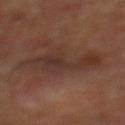Clinical impression:
No biopsy was performed on this lesion — it was imaged during a full skin examination and was not determined to be concerning.
Acquisition and patient details:
Automated tile analysis of the lesion measured a lesion area of about 15 mm², a shape eccentricity near 0.9, and a shape-asymmetry score of about 0.45 (0 = symmetric). A 15 mm close-up extracted from a 3D total-body photography capture. The subject is a male aged around 70. Longest diameter approximately 7 mm. This is a cross-polarized tile. From the mid back.